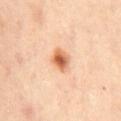  biopsy_status: not biopsied; imaged during a skin examination
  lesion_size:
    long_diameter_mm_approx: 3.0
  site: mid back
  image:
    source: total-body photography crop
    field_of_view_mm: 15
  automated_metrics:
    nevus_likeness_0_100: 100
    lesion_detection_confidence_0_100: 100
  patient:
    sex: female
    age_approx: 60